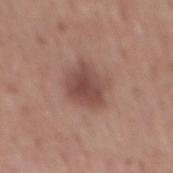biopsy status: catalogued during a skin exam; not biopsied | acquisition: ~15 mm tile from a whole-body skin photo | illumination: white-light illumination | patient: male, aged 53–57 | anatomic site: the mid back | diameter: ~4.5 mm (longest diameter).